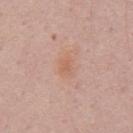Located on the chest.
A lesion tile, about 15 mm wide, cut from a 3D total-body photograph.
A male patient, in their mid-60s.
Automated image analysis of the tile measured a shape eccentricity near 0.65 and a symmetry-axis asymmetry near 0.25.
The lesion's longest dimension is about 2.5 mm.